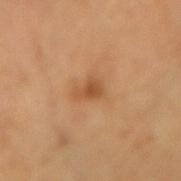Findings:
* biopsy status — imaged on a skin check; not biopsied
* acquisition — ~15 mm crop, total-body skin-cancer survey
* tile lighting — cross-polarized
* diameter — about 3 mm
* subject — female, aged 53 to 57
* location — the arm